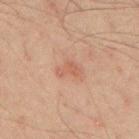This lesion was catalogued during total-body skin photography and was not selected for biopsy.
Cropped from a whole-body photographic skin survey; the tile spans about 15 mm.
Longest diameter approximately 3 mm.
An algorithmic analysis of the crop reported an automated nevus-likeness rating near 5 out of 100 and a detector confidence of about 100 out of 100 that the crop contains a lesion.
Located on the mid back.
This is a cross-polarized tile.
A male patient aged 43–47.